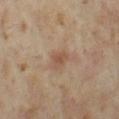follow-up=no biopsy performed (imaged during a skin exam) | tile lighting=cross-polarized illumination | image source=15 mm crop, total-body photography | patient=female, aged 33 to 37 | body site=the left thigh.